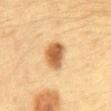Clinical impression: Recorded during total-body skin imaging; not selected for excision or biopsy. Background: The lesion is located on the front of the torso. This image is a 15 mm lesion crop taken from a total-body photograph. The subject is a female aged approximately 30.